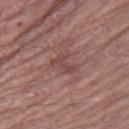Assessment: The lesion was photographed on a routine skin check and not biopsied; there is no pathology result. Image and clinical context: The patient is a male aged 63 to 67. The lesion is on the right thigh. Approximately 3.5 mm at its widest. Imaged with white-light lighting. A 15 mm close-up extracted from a 3D total-body photography capture. The lesion-visualizer software estimated a mean CIELAB color near L≈45 a*≈22 b*≈21 and a lesion–skin lightness drop of about 7.